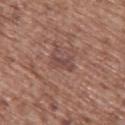follow-up — catalogued during a skin exam; not biopsied
image source — total-body-photography crop, ~15 mm field of view
subject — female, aged around 75
location — the upper back
lesion diameter — ~3 mm (longest diameter)
lighting — white-light